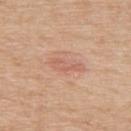Impression: The lesion was photographed on a routine skin check and not biopsied; there is no pathology result. Background: A male subject, aged approximately 80. Located on the back. A 15 mm close-up tile from a total-body photography series done for melanoma screening.